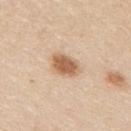Notes:
* follow-up · no biopsy performed (imaged during a skin exam)
* diameter · about 3.5 mm
* site · the upper back
* subject · male, aged 33 to 37
* lighting · white-light
* image source · 15 mm crop, total-body photography